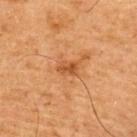Assessment: Part of a total-body skin-imaging series; this lesion was reviewed on a skin check and was not flagged for biopsy. Background: Automated tile analysis of the lesion measured an eccentricity of roughly 0.75. And it measured a mean CIELAB color near L≈43 a*≈23 b*≈36 and roughly 8 lightness units darker than nearby skin. The recorded lesion diameter is about 2.5 mm. From the back. Imaged with cross-polarized lighting. A male patient in their mid-60s. A roughly 15 mm field-of-view crop from a total-body skin photograph.